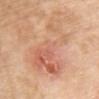| key | value |
|---|---|
| notes | no biopsy performed (imaged during a skin exam) |
| site | the chest |
| patient | female, aged 68–72 |
| image | ~15 mm crop, total-body skin-cancer survey |